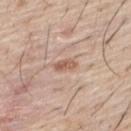Notes:
– lighting: white-light illumination
– subject: male, roughly 55 years of age
– diameter: ~3 mm (longest diameter)
– TBP lesion metrics: a footprint of about 3 mm², a shape eccentricity near 0.9, and two-axis asymmetry of about 0.2; an average lesion color of about L≈58 a*≈20 b*≈29 (CIELAB), roughly 11 lightness units darker than nearby skin, and a normalized lesion–skin contrast near 7.5
– location: the upper back
– image source: ~15 mm tile from a whole-body skin photo